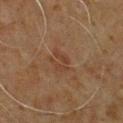Q: Was a biopsy performed?
A: total-body-photography surveillance lesion; no biopsy
Q: Lesion location?
A: the chest
Q: What are the patient's age and sex?
A: male, aged around 60
Q: How was the tile lit?
A: cross-polarized illumination
Q: What did automated image analysis measure?
A: a lesion area of about 3.5 mm², an eccentricity of roughly 0.8, and a shape-asymmetry score of about 0.4 (0 = symmetric); a border-irregularity rating of about 5.5/10, internal color variation of about 0 on a 0–10 scale, and radial color variation of about 0
Q: How was this image acquired?
A: ~15 mm tile from a whole-body skin photo
Q: Lesion size?
A: ~3 mm (longest diameter)